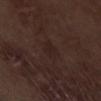The lesion was tiled from a total-body skin photograph and was not biopsied. The tile uses white-light illumination. The recorded lesion diameter is about 2.5 mm. This image is a 15 mm lesion crop taken from a total-body photograph. The subject is a male aged approximately 70. The lesion is located on the left thigh.